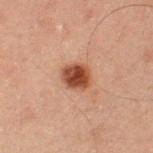Recorded during total-body skin imaging; not selected for excision or biopsy.
A male patient, aged approximately 70.
The lesion is on the left thigh.
The lesion's longest dimension is about 3 mm.
A 15 mm crop from a total-body photograph taken for skin-cancer surveillance.
An algorithmic analysis of the crop reported an area of roughly 6.5 mm², an eccentricity of roughly 0.55, and a symmetry-axis asymmetry near 0.15. It also reported an average lesion color of about L≈35 a*≈21 b*≈26 (CIELAB), a lesion–skin lightness drop of about 13, and a normalized border contrast of about 11.5. The analysis additionally found a border-irregularity rating of about 1.5/10, a within-lesion color-variation index near 3.5/10, and peripheral color asymmetry of about 1.
Imaged with cross-polarized lighting.